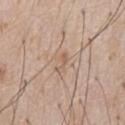Clinical impression:
Part of a total-body skin-imaging series; this lesion was reviewed on a skin check and was not flagged for biopsy.
Image and clinical context:
An algorithmic analysis of the crop reported a footprint of about 2.5 mm², an outline eccentricity of about 0.95 (0 = round, 1 = elongated), and a symmetry-axis asymmetry near 0.45. The software also gave border irregularity of about 5.5 on a 0–10 scale, a within-lesion color-variation index near 0/10, and a peripheral color-asymmetry measure near 0. On the chest. The patient is a male aged 58–62. Imaged with white-light lighting. A roughly 15 mm field-of-view crop from a total-body skin photograph. Measured at roughly 3 mm in maximum diameter.